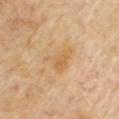Assessment: Captured during whole-body skin photography for melanoma surveillance; the lesion was not biopsied.